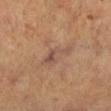Recorded during total-body skin imaging; not selected for excision or biopsy.
Located on the right lower leg.
A close-up tile cropped from a whole-body skin photograph, about 15 mm across.
A female patient, aged approximately 60.